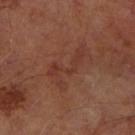Background:
A male subject, about 70 years old. The lesion is on the left forearm. This is a cross-polarized tile. The lesion's longest dimension is about 5.5 mm. A region of skin cropped from a whole-body photographic capture, roughly 15 mm wide.